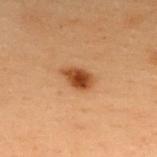Captured under cross-polarized illumination.
The lesion is located on the upper back.
A region of skin cropped from a whole-body photographic capture, roughly 15 mm wide.
A female patient in their 40s.
The lesion's longest dimension is about 3.5 mm.